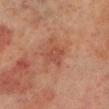follow-up — no biopsy performed (imaged during a skin exam); diameter — ~2.5 mm (longest diameter); illumination — cross-polarized illumination; patient — male, aged 68 to 72; acquisition — ~15 mm tile from a whole-body skin photo; anatomic site — the right lower leg.